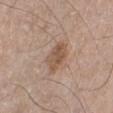biopsy status: catalogued during a skin exam; not biopsied
lesion diameter: ~3.5 mm (longest diameter)
subject: male, aged approximately 70
illumination: white-light illumination
imaging modality: ~15 mm tile from a whole-body skin photo
body site: the left lower leg